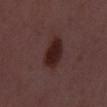Image and clinical context:
Captured under white-light illumination. The recorded lesion diameter is about 4.5 mm. The lesion-visualizer software estimated a border-irregularity rating of about 2/10 and peripheral color asymmetry of about 1. It also reported an automated nevus-likeness rating near 95 out of 100 and a lesion-detection confidence of about 100/100. The subject is a male in their 50s. This image is a 15 mm lesion crop taken from a total-body photograph.